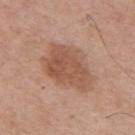Q: Was a biopsy performed?
A: imaged on a skin check; not biopsied
Q: Where on the body is the lesion?
A: the back
Q: How was this image acquired?
A: ~15 mm tile from a whole-body skin photo
Q: Lesion size?
A: about 7 mm
Q: What did automated image analysis measure?
A: a classifier nevus-likeness of about 25/100
Q: Who is the patient?
A: male, aged around 55
Q: What lighting was used for the tile?
A: white-light illumination